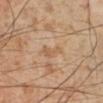follow-up: catalogued during a skin exam; not biopsied
body site: the left lower leg
subject: male, aged approximately 55
image source: ~15 mm tile from a whole-body skin photo